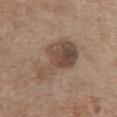biopsy status: no biopsy performed (imaged during a skin exam); imaging modality: 15 mm crop, total-body photography; anatomic site: the chest; patient: female, aged 63–67; tile lighting: white-light.